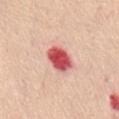Acquisition and patient details: Longest diameter approximately 4 mm. The tile uses white-light illumination. The lesion-visualizer software estimated a border-irregularity index near 1.5/10 and a within-lesion color-variation index near 5.5/10. It also reported a classifier nevus-likeness of about 0/100 and a detector confidence of about 100 out of 100 that the crop contains a lesion. A 15 mm close-up extracted from a 3D total-body photography capture. A female patient, aged approximately 45. The lesion is located on the abdomen.